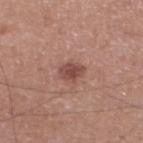patient:
  sex: male
  age_approx: 50
automated_metrics:
  eccentricity: 0.6
  shape_asymmetry: 0.2
  cielab_L: 47
  cielab_a: 23
  cielab_b: 25
  vs_skin_darker_L: 11.0
  vs_skin_contrast_norm: 8.0
  color_variation_0_10: 1.5
  peripheral_color_asymmetry: 0.5
image:
  source: total-body photography crop
  field_of_view_mm: 15
site: left lower leg
lighting: white-light